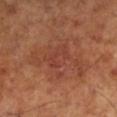Clinical summary: About 7.5 mm across. Captured under cross-polarized illumination. Automated image analysis of the tile measured a border-irregularity index near 5.5/10, a within-lesion color-variation index near 4.5/10, and peripheral color asymmetry of about 1.5. And it measured an automated nevus-likeness rating near 5 out of 100 and a detector confidence of about 100 out of 100 that the crop contains a lesion. A region of skin cropped from a whole-body photographic capture, roughly 15 mm wide. From the left lower leg. A male subject, aged approximately 65.